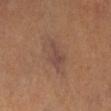Clinical impression: This lesion was catalogued during total-body skin photography and was not selected for biopsy. Background: The patient is a female aged around 65. The recorded lesion diameter is about 5 mm. Cropped from a total-body skin-imaging series; the visible field is about 15 mm. On the left lower leg.